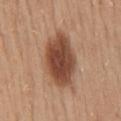| key | value |
|---|---|
| biopsy status | catalogued during a skin exam; not biopsied |
| image-analysis metrics | a border-irregularity rating of about 2/10 and radial color variation of about 1.5 |
| body site | the back |
| patient | female, aged 38 to 42 |
| tile lighting | white-light illumination |
| acquisition | total-body-photography crop, ~15 mm field of view |